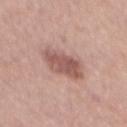Background:
A male patient, approximately 55 years of age. Automated image analysis of the tile measured a lesion area of about 13 mm² and a shape eccentricity near 0.8. The software also gave radial color variation of about 1.5. A roughly 15 mm field-of-view crop from a total-body skin photograph. On the mid back. The recorded lesion diameter is about 5.5 mm.